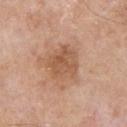<lesion>
  <biopsy_status>not biopsied; imaged during a skin examination</biopsy_status>
  <automated_metrics>
    <area_mm2_approx>12.0</area_mm2_approx>
    <eccentricity>0.4</eccentricity>
    <shape_asymmetry>0.2</shape_asymmetry>
  </automated_metrics>
  <site>upper back</site>
  <patient>
    <sex>male</sex>
    <age_approx>55</age_approx>
  </patient>
  <lighting>white-light</lighting>
  <image>
    <source>total-body photography crop</source>
    <field_of_view_mm>15</field_of_view_mm>
  </image>
</lesion>